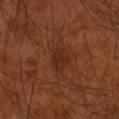Impression: Recorded during total-body skin imaging; not selected for excision or biopsy. Background: The subject is a male approximately 50 years of age. On the arm. This image is a 15 mm lesion crop taken from a total-body photograph.